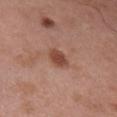Notes:
- tile lighting · white-light illumination
- lesion size · ≈2.5 mm
- patient · male, aged around 55
- anatomic site · the right upper arm
- automated metrics · a footprint of about 4 mm², a shape eccentricity near 0.75, and a shape-asymmetry score of about 0.2 (0 = symmetric); an automated nevus-likeness rating near 80 out of 100 and a detector confidence of about 100 out of 100 that the crop contains a lesion
- image source · total-body-photography crop, ~15 mm field of view